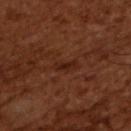Captured during whole-body skin photography for melanoma surveillance; the lesion was not biopsied. About 3 mm across. Cropped from a whole-body photographic skin survey; the tile spans about 15 mm. Imaged with cross-polarized lighting. The subject is a male about 65 years old.